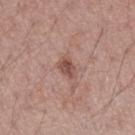follow-up: imaged on a skin check; not biopsied
location: the arm
patient: female, aged around 55
image: ~15 mm crop, total-body skin-cancer survey
lighting: white-light illumination
lesion diameter: ~2.5 mm (longest diameter)
image-analysis metrics: a lesion area of about 4 mm², an outline eccentricity of about 0.7 (0 = round, 1 = elongated), and a shape-asymmetry score of about 0.25 (0 = symmetric); an average lesion color of about L≈50 a*≈22 b*≈25 (CIELAB), about 11 CIELAB-L* units darker than the surrounding skin, and a normalized border contrast of about 7.5; an automated nevus-likeness rating near 75 out of 100 and a lesion-detection confidence of about 100/100